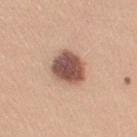The lesion was photographed on a routine skin check and not biopsied; there is no pathology result.
A female patient aged around 20.
The lesion is on the right upper arm.
A region of skin cropped from a whole-body photographic capture, roughly 15 mm wide.
Imaged with white-light lighting.